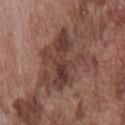Imaged during a routine full-body skin examination; the lesion was not biopsied and no histopathology is available.
A male subject, roughly 75 years of age.
A 15 mm close-up extracted from a 3D total-body photography capture.
Located on the chest.